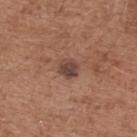{
  "biopsy_status": "not biopsied; imaged during a skin examination",
  "site": "upper back",
  "automated_metrics": {
    "border_irregularity_0_10": 1.0,
    "color_variation_0_10": 3.0,
    "peripheral_color_asymmetry": 1.0
  },
  "image": {
    "source": "total-body photography crop",
    "field_of_view_mm": 15
  },
  "lighting": "white-light",
  "patient": {
    "sex": "male",
    "age_approx": 75
  }
}